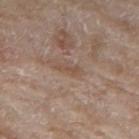• illumination — white-light illumination
• image source — ~15 mm crop, total-body skin-cancer survey
• subject — female, approximately 55 years of age
• location — the left forearm
• image-analysis metrics — an area of roughly 2.5 mm², an outline eccentricity of about 0.95 (0 = round, 1 = elongated), and a symmetry-axis asymmetry near 0.5; a detector confidence of about 95 out of 100 that the crop contains a lesion